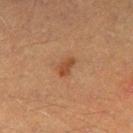The patient is a male roughly 40 years of age.
From the right thigh.
Cropped from a total-body skin-imaging series; the visible field is about 15 mm.